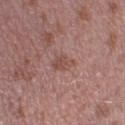Assessment:
This lesion was catalogued during total-body skin photography and was not selected for biopsy.
Image and clinical context:
The lesion is located on the left lower leg. This is a white-light tile. A roughly 15 mm field-of-view crop from a total-body skin photograph. A male patient, aged 38–42. The total-body-photography lesion software estimated border irregularity of about 4 on a 0–10 scale and peripheral color asymmetry of about 0.5.